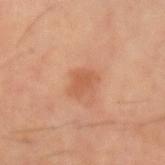<case>
  <biopsy_status>not biopsied; imaged during a skin examination</biopsy_status>
  <lesion_size>
    <long_diameter_mm_approx>3.0</long_diameter_mm_approx>
  </lesion_size>
  <lighting>cross-polarized</lighting>
  <image>
    <source>total-body photography crop</source>
    <field_of_view_mm>15</field_of_view_mm>
  </image>
  <patient>
    <sex>male</sex>
    <age_approx>60</age_approx>
  </patient>
  <site>left forearm</site>
</case>